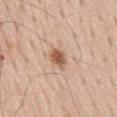Q: Was this lesion biopsied?
A: catalogued during a skin exam; not biopsied
Q: What lighting was used for the tile?
A: white-light
Q: What is the lesion's diameter?
A: ~2.5 mm (longest diameter)
Q: What kind of image is this?
A: ~15 mm crop, total-body skin-cancer survey
Q: What are the patient's age and sex?
A: male, about 60 years old
Q: What is the anatomic site?
A: the mid back